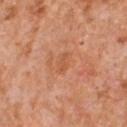Q: Was this lesion biopsied?
A: no biopsy performed (imaged during a skin exam)
Q: How large is the lesion?
A: ≈2.5 mm
Q: Automated lesion metrics?
A: an area of roughly 3 mm², an eccentricity of roughly 0.85, and a symmetry-axis asymmetry near 0.2; a mean CIELAB color near L≈56 a*≈27 b*≈38, a lesion–skin lightness drop of about 6, and a lesion-to-skin contrast of about 5 (normalized; higher = more distinct)
Q: How was this image acquired?
A: 15 mm crop, total-body photography
Q: What are the patient's age and sex?
A: male, roughly 55 years of age
Q: Lesion location?
A: the chest
Q: What lighting was used for the tile?
A: white-light illumination